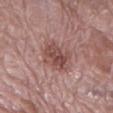This lesion was catalogued during total-body skin photography and was not selected for biopsy. A 15 mm crop from a total-body photograph taken for skin-cancer surveillance. A female patient aged approximately 70. Captured under white-light illumination. From the left thigh. The recorded lesion diameter is about 4 mm.